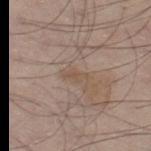Assessment: The lesion was tiled from a total-body skin photograph and was not biopsied. Acquisition and patient details: The lesion is located on the right thigh. A male patient, aged approximately 55. About 2.5 mm across. The lesion-visualizer software estimated a mean CIELAB color near L≈51 a*≈15 b*≈27, a lesion–skin lightness drop of about 6, and a normalized lesion–skin contrast near 5. A close-up tile cropped from a whole-body skin photograph, about 15 mm across. Captured under white-light illumination.